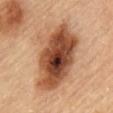follow-up: imaged on a skin check; not biopsied | tile lighting: cross-polarized illumination | patient: male, in their mid- to late 80s or older | image-analysis metrics: an area of roughly 38 mm², an eccentricity of roughly 0.85, and a shape-asymmetry score of about 0.2 (0 = symmetric); a mean CIELAB color near L≈48 a*≈23 b*≈33, roughly 18 lightness units darker than nearby skin, and a normalized border contrast of about 12.5 | imaging modality: 15 mm crop, total-body photography | body site: the chest.